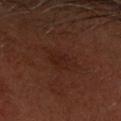| key | value |
|---|---|
| workup | total-body-photography surveillance lesion; no biopsy |
| patient | male, about 60 years old |
| lesion diameter | ~3.5 mm (longest diameter) |
| image | 15 mm crop, total-body photography |
| site | the head or neck |